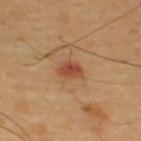Q: Was a biopsy performed?
A: imaged on a skin check; not biopsied
Q: What kind of image is this?
A: ~15 mm tile from a whole-body skin photo
Q: Illumination type?
A: cross-polarized
Q: What is the anatomic site?
A: the upper back
Q: Patient demographics?
A: male, aged 63–67